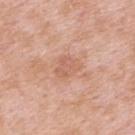Findings:
- follow-up: total-body-photography surveillance lesion; no biopsy
- diameter: ~3 mm (longest diameter)
- imaging modality: ~15 mm tile from a whole-body skin photo
- tile lighting: white-light illumination
- site: the left upper arm
- subject: male, aged approximately 55
- automated lesion analysis: an average lesion color of about L≈61 a*≈23 b*≈31 (CIELAB) and a normalized lesion–skin contrast near 5